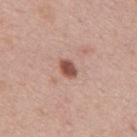No biopsy was performed on this lesion — it was imaged during a full skin examination and was not determined to be concerning. The lesion is on the mid back. A region of skin cropped from a whole-body photographic capture, roughly 15 mm wide. The subject is a male roughly 45 years of age.